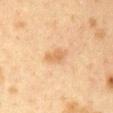Impression:
No biopsy was performed on this lesion — it was imaged during a full skin examination and was not determined to be concerning.
Clinical summary:
Captured under cross-polarized illumination. A close-up tile cropped from a whole-body skin photograph, about 15 mm across. The lesion-visualizer software estimated a footprint of about 3.5 mm² and a shape eccentricity near 0.85. And it measured a normalized border contrast of about 6. And it measured border irregularity of about 2.5 on a 0–10 scale, internal color variation of about 0.5 on a 0–10 scale, and peripheral color asymmetry of about 0. And it measured a classifier nevus-likeness of about 20/100 and a lesion-detection confidence of about 100/100. A female subject aged around 40. On the chest.